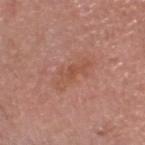{"biopsy_status": "not biopsied; imaged during a skin examination", "image": {"source": "total-body photography crop", "field_of_view_mm": 15}, "patient": {"sex": "male", "age_approx": 65}, "site": "head or neck"}